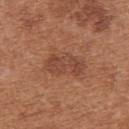Q: Is there a histopathology result?
A: no biopsy performed (imaged during a skin exam)
Q: Illumination type?
A: white-light illumination
Q: Where on the body is the lesion?
A: the upper back
Q: Who is the patient?
A: female, about 40 years old
Q: How large is the lesion?
A: ~5 mm (longest diameter)
Q: What is the imaging modality?
A: ~15 mm tile from a whole-body skin photo
Q: Automated lesion metrics?
A: a within-lesion color-variation index near 2.5/10 and peripheral color asymmetry of about 1; a nevus-likeness score of about 25/100 and a lesion-detection confidence of about 100/100